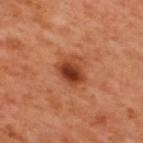Case summary:
- notes · imaged on a skin check; not biopsied
- tile lighting · cross-polarized illumination
- TBP lesion metrics · an average lesion color of about L≈42 a*≈29 b*≈36 (CIELAB) and a lesion–skin lightness drop of about 13; border irregularity of about 2.5 on a 0–10 scale, a within-lesion color-variation index near 8/10, and peripheral color asymmetry of about 3; a classifier nevus-likeness of about 95/100 and a detector confidence of about 100 out of 100 that the crop contains a lesion
- imaging modality · total-body-photography crop, ~15 mm field of view
- patient · male, about 50 years old
- anatomic site · the upper back